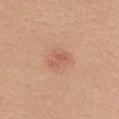Notes:
• follow-up: no biopsy performed (imaged during a skin exam)